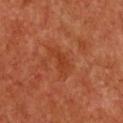acquisition=~15 mm tile from a whole-body skin photo
subject=female, in their mid-60s
body site=the chest
tile lighting=cross-polarized illumination
TBP lesion metrics=an average lesion color of about L≈40 a*≈30 b*≈37 (CIELAB), a lesion–skin lightness drop of about 6, and a normalized border contrast of about 5.5; border irregularity of about 5.5 on a 0–10 scale, a within-lesion color-variation index near 0.5/10, and a peripheral color-asymmetry measure near 0; a classifier nevus-likeness of about 0/100 and a detector confidence of about 100 out of 100 that the crop contains a lesion